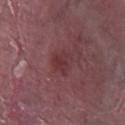Imaged with white-light lighting. From the right lower leg. The recorded lesion diameter is about 3 mm. This image is a 15 mm lesion crop taken from a total-body photograph. A male subject, roughly 40 years of age.